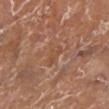Findings:
- notes: imaged on a skin check; not biopsied
- image-analysis metrics: a footprint of about 3.5 mm² and an eccentricity of roughly 0.8
- anatomic site: the leg
- subject: female, aged around 75
- image: total-body-photography crop, ~15 mm field of view
- lesion size: ≈2.5 mm
- illumination: white-light